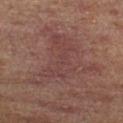Q: Was this lesion biopsied?
A: no biopsy performed (imaged during a skin exam)
Q: Where on the body is the lesion?
A: the right lower leg
Q: Who is the patient?
A: male, aged 63–67
Q: How was this image acquired?
A: total-body-photography crop, ~15 mm field of view